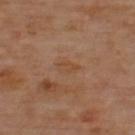Assessment:
This lesion was catalogued during total-body skin photography and was not selected for biopsy.
Background:
Measured at roughly 3 mm in maximum diameter. A 15 mm crop from a total-body photograph taken for skin-cancer surveillance. Located on the upper back. This is a cross-polarized tile. An algorithmic analysis of the crop reported an eccentricity of roughly 0.8. The analysis additionally found a lesion color around L≈48 a*≈20 b*≈33 in CIELAB and roughly 5 lightness units darker than nearby skin. It also reported border irregularity of about 2.5 on a 0–10 scale, internal color variation of about 1.5 on a 0–10 scale, and a peripheral color-asymmetry measure near 0.5. The software also gave a nevus-likeness score of about 0/100 and a detector confidence of about 100 out of 100 that the crop contains a lesion.